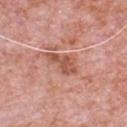{
  "biopsy_status": "not biopsied; imaged during a skin examination",
  "image": {
    "source": "total-body photography crop",
    "field_of_view_mm": 15
  },
  "patient": {
    "sex": "male",
    "age_approx": 80
  },
  "site": "chest"
}